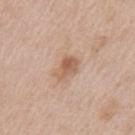Findings:
– workup: catalogued during a skin exam; not biopsied
– subject: female, aged approximately 40
– anatomic site: the left upper arm
– acquisition: ~15 mm tile from a whole-body skin photo
– size: about 3 mm
– tile lighting: white-light illumination